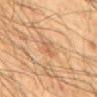Findings:
• biopsy status: catalogued during a skin exam; not biopsied
• imaging modality: total-body-photography crop, ~15 mm field of view
• image-analysis metrics: a mean CIELAB color near L≈48 a*≈18 b*≈31, roughly 6 lightness units darker than nearby skin, and a normalized lesion–skin contrast near 4.5; a border-irregularity rating of about 4/10, a color-variation rating of about 2/10, and radial color variation of about 1; an automated nevus-likeness rating near 0 out of 100 and lesion-presence confidence of about 80/100
• size: ~3 mm (longest diameter)
• tile lighting: cross-polarized
• subject: male, aged approximately 65
• location: the mid back